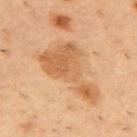  biopsy_status: not biopsied; imaged during a skin examination